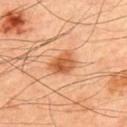Assessment:
This lesion was catalogued during total-body skin photography and was not selected for biopsy.
Acquisition and patient details:
A close-up tile cropped from a whole-body skin photograph, about 15 mm across. This is a cross-polarized tile. The patient is a male aged 48 to 52. The lesion is on the upper back. About 3.5 mm across. Automated image analysis of the tile measured an outline eccentricity of about 0.7 (0 = round, 1 = elongated) and a shape-asymmetry score of about 0.3 (0 = symmetric). It also reported a mean CIELAB color near L≈58 a*≈28 b*≈41 and a lesion–skin lightness drop of about 13. The software also gave border irregularity of about 2.5 on a 0–10 scale, internal color variation of about 5 on a 0–10 scale, and radial color variation of about 1.5.